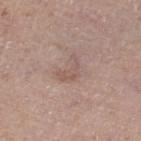Recorded during total-body skin imaging; not selected for excision or biopsy. A female subject aged 63–67. A 15 mm close-up tile from a total-body photography series done for melanoma screening. Located on the left thigh. This is a white-light tile. The total-body-photography lesion software estimated an outline eccentricity of about 0.75 (0 = round, 1 = elongated) and a symmetry-axis asymmetry near 0.6. The software also gave a lesion color around L≈55 a*≈17 b*≈23 in CIELAB. And it measured a border-irregularity index near 7/10 and a peripheral color-asymmetry measure near 0.5.